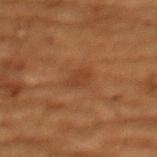Imaged during a routine full-body skin examination; the lesion was not biopsied and no histopathology is available.